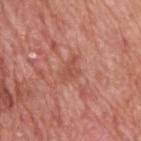Case summary:
• size — ~3.5 mm (longest diameter)
• site — the back
• lighting — white-light illumination
• patient — male, aged 58–62
• imaging modality — ~15 mm crop, total-body skin-cancer survey
• TBP lesion metrics — an outline eccentricity of about 0.7 (0 = round, 1 = elongated) and a symmetry-axis asymmetry near 0.35; a mean CIELAB color near L≈53 a*≈27 b*≈30, roughly 6 lightness units darker than nearby skin, and a normalized lesion–skin contrast near 5; a border-irregularity rating of about 3.5/10, a color-variation rating of about 2/10, and radial color variation of about 1; a classifier nevus-likeness of about 0/100 and a detector confidence of about 100 out of 100 that the crop contains a lesion